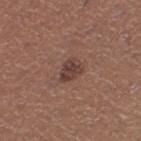The lesion was tiled from a total-body skin photograph and was not biopsied.
A 15 mm close-up extracted from a 3D total-body photography capture.
A female patient aged around 40.
The lesion is located on the left thigh.
Automated tile analysis of the lesion measured an average lesion color of about L≈40 a*≈19 b*≈22 (CIELAB) and a lesion–skin lightness drop of about 9. It also reported a border-irregularity index near 2/10, a color-variation rating of about 2.5/10, and radial color variation of about 1.
This is a white-light tile.